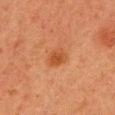{
  "biopsy_status": "not biopsied; imaged during a skin examination",
  "automated_metrics": {
    "cielab_L": 43,
    "cielab_a": 25,
    "cielab_b": 36,
    "vs_skin_darker_L": 8.0,
    "border_irregularity_0_10": 1.5,
    "peripheral_color_asymmetry": 1.0
  },
  "patient": {
    "sex": "female",
    "age_approx": 40
  },
  "image": {
    "source": "total-body photography crop",
    "field_of_view_mm": 15
  },
  "site": "front of the torso",
  "lighting": "cross-polarized",
  "lesion_size": {
    "long_diameter_mm_approx": 2.5
  }
}